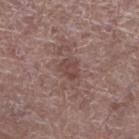{"biopsy_status": "not biopsied; imaged during a skin examination", "site": "left lower leg", "lighting": "white-light", "image": {"source": "total-body photography crop", "field_of_view_mm": 15}, "automated_metrics": {"shape_asymmetry": 0.2, "vs_skin_darker_L": 7.0, "border_irregularity_0_10": 2.0, "peripheral_color_asymmetry": 0.5, "nevus_likeness_0_100": 0, "lesion_detection_confidence_0_100": 100}, "lesion_size": {"long_diameter_mm_approx": 2.5}, "patient": {"sex": "male", "age_approx": 70}}